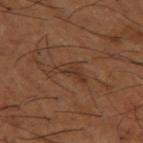No biopsy was performed on this lesion — it was imaged during a full skin examination and was not determined to be concerning. Located on the left thigh. A roughly 15 mm field-of-view crop from a total-body skin photograph. About 4 mm across. A male subject, in their mid- to late 60s.